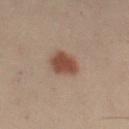Clinical impression:
No biopsy was performed on this lesion — it was imaged during a full skin examination and was not determined to be concerning.
Context:
A female patient, about 30 years old. A roughly 15 mm field-of-view crop from a total-body skin photograph. This is a cross-polarized tile. On the left thigh.